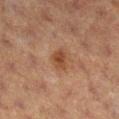workup: total-body-photography surveillance lesion; no biopsy | site: the right lower leg | patient: female, roughly 40 years of age | tile lighting: cross-polarized | imaging modality: ~15 mm tile from a whole-body skin photo | diameter: about 3 mm.